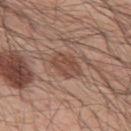Part of a total-body skin-imaging series; this lesion was reviewed on a skin check and was not flagged for biopsy.
A male subject, roughly 55 years of age.
Imaged with white-light lighting.
A lesion tile, about 15 mm wide, cut from a 3D total-body photograph.
The lesion's longest dimension is about 4 mm.
Automated image analysis of the tile measured an outline eccentricity of about 0.7 (0 = round, 1 = elongated) and two-axis asymmetry of about 0.2. The analysis additionally found about 9 CIELAB-L* units darker than the surrounding skin and a normalized lesion–skin contrast near 6.5.
Located on the upper back.